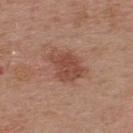follow-up — total-body-photography surveillance lesion; no biopsy
subject — male, aged 53 to 57
acquisition — 15 mm crop, total-body photography
site — the upper back
lighting — white-light illumination
diameter — about 4.5 mm
automated lesion analysis — a lesion–skin lightness drop of about 10 and a lesion-to-skin contrast of about 7.5 (normalized; higher = more distinct); border irregularity of about 3.5 on a 0–10 scale, internal color variation of about 2.5 on a 0–10 scale, and peripheral color asymmetry of about 1